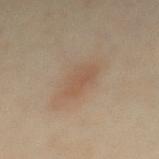Clinical impression: The lesion was photographed on a routine skin check and not biopsied; there is no pathology result. Context: Measured at roughly 5 mm in maximum diameter. A female patient roughly 35 years of age. A roughly 15 mm field-of-view crop from a total-body skin photograph. An algorithmic analysis of the crop reported an average lesion color of about L≈51 a*≈16 b*≈28 (CIELAB), about 6 CIELAB-L* units darker than the surrounding skin, and a normalized border contrast of about 5. The software also gave border irregularity of about 3 on a 0–10 scale and a color-variation rating of about 2.5/10. The analysis additionally found an automated nevus-likeness rating near 50 out of 100 and lesion-presence confidence of about 100/100. The lesion is located on the mid back.